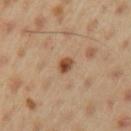{"biopsy_status": "not biopsied; imaged during a skin examination", "image": {"source": "total-body photography crop", "field_of_view_mm": 15}, "patient": {"sex": "male", "age_approx": 45}, "site": "left upper arm"}